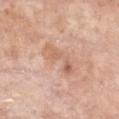biopsy status = no biopsy performed (imaged during a skin exam); site = the chest; subject = female, aged 73–77; imaging modality = 15 mm crop, total-body photography.